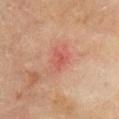| key | value |
|---|---|
| workup | no biopsy performed (imaged during a skin exam) |
| subject | female, aged approximately 50 |
| illumination | cross-polarized illumination |
| image source | ~15 mm crop, total-body skin-cancer survey |
| anatomic site | the chest |
| lesion diameter | ~3 mm (longest diameter) |